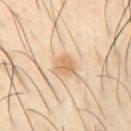Background:
On the left upper arm. The recorded lesion diameter is about 2.5 mm. A roughly 15 mm field-of-view crop from a total-body skin photograph. This is a cross-polarized tile. The patient is a male about 40 years old.